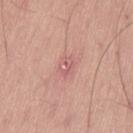diameter — about 2.5 mm
subject — male, aged 53 to 57
location — the left thigh
image source — total-body-photography crop, ~15 mm field of view
illumination — white-light